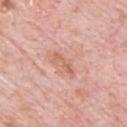follow-up — imaged on a skin check; not biopsied
lighting — white-light
location — the chest
imaging modality — ~15 mm tile from a whole-body skin photo
lesion diameter — ~3.5 mm (longest diameter)
patient — male, aged 78–82